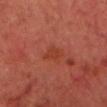Impression: No biopsy was performed on this lesion — it was imaged during a full skin examination and was not determined to be concerning. Acquisition and patient details: On the head or neck. A male subject, aged 68–72. This is a cross-polarized tile. A region of skin cropped from a whole-body photographic capture, roughly 15 mm wide. Automated image analysis of the tile measured an area of roughly 3.5 mm², an eccentricity of roughly 0.7, and a symmetry-axis asymmetry near 0.35. It also reported border irregularity of about 3 on a 0–10 scale, a within-lesion color-variation index near 1/10, and peripheral color asymmetry of about 0.5.